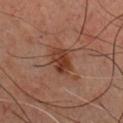Imaged during a routine full-body skin examination; the lesion was not biopsied and no histopathology is available. A male subject, aged around 70. The tile uses cross-polarized illumination. The lesion is located on the front of the torso. The total-body-photography lesion software estimated an area of roughly 7.5 mm², an eccentricity of roughly 0.65, and two-axis asymmetry of about 0.2. It also reported a border-irregularity rating of about 2.5/10 and a within-lesion color-variation index near 4.5/10. A lesion tile, about 15 mm wide, cut from a 3D total-body photograph.